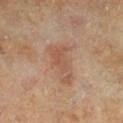Impression:
The lesion was tiled from a total-body skin photograph and was not biopsied.
Context:
Longest diameter approximately 5 mm. Automated tile analysis of the lesion measured an area of roughly 9.5 mm², an outline eccentricity of about 0.9 (0 = round, 1 = elongated), and a shape-asymmetry score of about 0.45 (0 = symmetric). And it measured a border-irregularity rating of about 5.5/10 and a within-lesion color-variation index near 2.5/10. The analysis additionally found a nevus-likeness score of about 0/100 and a lesion-detection confidence of about 100/100. The tile uses cross-polarized illumination. The subject is a male in their mid- to late 60s. A region of skin cropped from a whole-body photographic capture, roughly 15 mm wide. Located on the left lower leg.